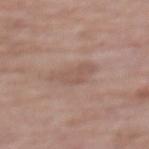This lesion was catalogued during total-body skin photography and was not selected for biopsy.
The patient is a female aged approximately 75.
The lesion's longest dimension is about 4 mm.
The lesion is on the mid back.
The lesion-visualizer software estimated a lesion area of about 6.5 mm², an eccentricity of roughly 0.85, and a shape-asymmetry score of about 0.4 (0 = symmetric). It also reported about 7 CIELAB-L* units darker than the surrounding skin and a lesion-to-skin contrast of about 5 (normalized; higher = more distinct). It also reported an automated nevus-likeness rating near 0 out of 100.
The tile uses white-light illumination.
Cropped from a total-body skin-imaging series; the visible field is about 15 mm.